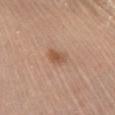Impression:
Recorded during total-body skin imaging; not selected for excision or biopsy.
Clinical summary:
A lesion tile, about 15 mm wide, cut from a 3D total-body photograph. A female patient, aged around 65. On the chest.